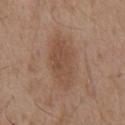Assessment: The lesion was tiled from a total-body skin photograph and was not biopsied. Image and clinical context: The patient is a male approximately 55 years of age. The lesion is on the abdomen. Longest diameter approximately 6 mm. A 15 mm close-up extracted from a 3D total-body photography capture. An algorithmic analysis of the crop reported an eccentricity of roughly 0.85 and a symmetry-axis asymmetry near 0.2. And it measured a mean CIELAB color near L≈49 a*≈18 b*≈29, about 7 CIELAB-L* units darker than the surrounding skin, and a lesion-to-skin contrast of about 5.5 (normalized; higher = more distinct). It also reported internal color variation of about 2.5 on a 0–10 scale and a peripheral color-asymmetry measure near 1. The software also gave a nevus-likeness score of about 45/100 and lesion-presence confidence of about 100/100. Captured under white-light illumination.